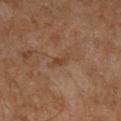Assessment:
Captured during whole-body skin photography for melanoma surveillance; the lesion was not biopsied.
Acquisition and patient details:
Located on the right lower leg. The recorded lesion diameter is about 3.5 mm. Captured under cross-polarized illumination. This image is a 15 mm lesion crop taken from a total-body photograph. A male patient approximately 60 years of age.